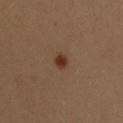Q: Is there a histopathology result?
A: total-body-photography surveillance lesion; no biopsy
Q: Automated lesion metrics?
A: a footprint of about 3 mm², an outline eccentricity of about 0.4 (0 = round, 1 = elongated), and a symmetry-axis asymmetry near 0.3; a mean CIELAB color near L≈35 a*≈19 b*≈30, roughly 10 lightness units darker than nearby skin, and a normalized lesion–skin contrast near 9.5
Q: Lesion location?
A: the upper back
Q: Lesion size?
A: ≈2 mm
Q: What are the patient's age and sex?
A: male, aged 28–32
Q: How was this image acquired?
A: ~15 mm crop, total-body skin-cancer survey
Q: Illumination type?
A: cross-polarized illumination